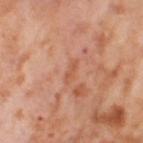The lesion was tiled from a total-body skin photograph and was not biopsied.
This image is a 15 mm lesion crop taken from a total-body photograph.
The subject is a female about 55 years old.
The lesion is on the right thigh.